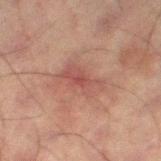Clinical impression: The lesion was tiled from a total-body skin photograph and was not biopsied. Context: A male patient, in their 60s. From the left thigh. A close-up tile cropped from a whole-body skin photograph, about 15 mm across.